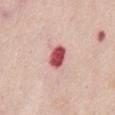Impression: Recorded during total-body skin imaging; not selected for excision or biopsy. Acquisition and patient details: The lesion is on the front of the torso. A region of skin cropped from a whole-body photographic capture, roughly 15 mm wide. A female patient in their mid-60s.